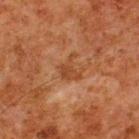Notes:
– notes — imaged on a skin check; not biopsied
– location — the back
– acquisition — ~15 mm tile from a whole-body skin photo
– subject — male, aged 78–82